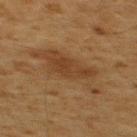The lesion was photographed on a routine skin check and not biopsied; there is no pathology result.